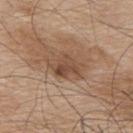Captured during whole-body skin photography for melanoma surveillance; the lesion was not biopsied. A male patient, approximately 75 years of age. Longest diameter approximately 5 mm. A 15 mm close-up extracted from a 3D total-body photography capture. From the upper back. Captured under white-light illumination.